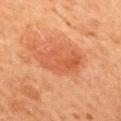follow-up: no biopsy performed (imaged during a skin exam) | image source: 15 mm crop, total-body photography | patient: female, aged around 50 | site: the upper back.